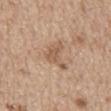Q: Is there a histopathology result?
A: catalogued during a skin exam; not biopsied
Q: How was this image acquired?
A: 15 mm crop, total-body photography
Q: Illumination type?
A: white-light
Q: What is the lesion's diameter?
A: about 4.5 mm
Q: Who is the patient?
A: male, in their 70s
Q: Lesion location?
A: the mid back